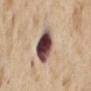patient: female, about 55 years old | anatomic site: the back | image: 15 mm crop, total-body photography | automated metrics: a footprint of about 13 mm² and a shape-asymmetry score of about 0.15 (0 = symmetric); a lesion color around L≈45 a*≈19 b*≈19 in CIELAB, about 25 CIELAB-L* units darker than the surrounding skin, and a normalized lesion–skin contrast near 18; a color-variation rating of about 10/10; a nevus-likeness score of about 85/100 and a detector confidence of about 100 out of 100 that the crop contains a lesion | lesion diameter: about 4.5 mm.